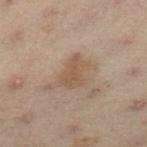Impression:
Part of a total-body skin-imaging series; this lesion was reviewed on a skin check and was not flagged for biopsy.
Clinical summary:
A female subject aged around 55. Captured under cross-polarized illumination. The lesion is on the left lower leg. A close-up tile cropped from a whole-body skin photograph, about 15 mm across.